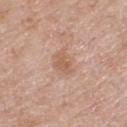Findings:
- biopsy status · catalogued during a skin exam; not biopsied